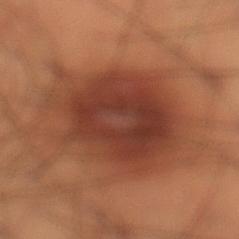From the left lower leg. A region of skin cropped from a whole-body photographic capture, roughly 15 mm wide. A male patient aged 48–52.